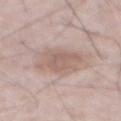{"site": "front of the torso", "patient": {"sex": "male", "age_approx": 75}, "image": {"source": "total-body photography crop", "field_of_view_mm": 15}, "lighting": "white-light"}